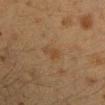Q: Was a biopsy performed?
A: total-body-photography surveillance lesion; no biopsy
Q: What is the anatomic site?
A: the right upper arm
Q: How large is the lesion?
A: ~2.5 mm (longest diameter)
Q: How was this image acquired?
A: total-body-photography crop, ~15 mm field of view
Q: What are the patient's age and sex?
A: female, aged 38 to 42
Q: Illumination type?
A: cross-polarized illumination